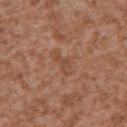<case>
<biopsy_status>not biopsied; imaged during a skin examination</biopsy_status>
</case>